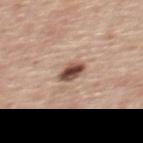No biopsy was performed on this lesion — it was imaged during a full skin examination and was not determined to be concerning.
Located on the upper back.
A male patient approximately 60 years of age.
This image is a 15 mm lesion crop taken from a total-body photograph.
Approximately 3 mm at its widest.
The tile uses white-light illumination.